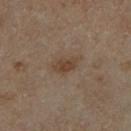Part of a total-body skin-imaging series; this lesion was reviewed on a skin check and was not flagged for biopsy.
Cropped from a total-body skin-imaging series; the visible field is about 15 mm.
The recorded lesion diameter is about 3 mm.
A male patient, aged around 65.
The lesion is located on the right lower leg.
Imaged with cross-polarized lighting.